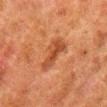Case summary:
– biopsy status: total-body-photography surveillance lesion; no biopsy
– size: ~4 mm (longest diameter)
– patient: male, aged around 80
– image source: 15 mm crop, total-body photography
– location: the right lower leg